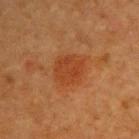Impression:
No biopsy was performed on this lesion — it was imaged during a full skin examination and was not determined to be concerning.
Context:
The lesion is located on the back. A 15 mm close-up tile from a total-body photography series done for melanoma screening. A female patient aged approximately 40. This is a cross-polarized tile. The lesion's longest dimension is about 3.5 mm.